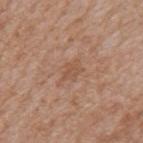workup: imaged on a skin check; not biopsied | automated lesion analysis: a footprint of about 3.5 mm², a shape eccentricity near 0.7, and a shape-asymmetry score of about 0.4 (0 = symmetric) | illumination: white-light illumination | location: the mid back | imaging modality: 15 mm crop, total-body photography | size: ≈2.5 mm | patient: male, approximately 65 years of age.